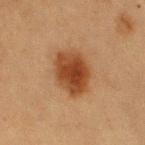Impression:
Recorded during total-body skin imaging; not selected for excision or biopsy.
Clinical summary:
A 15 mm close-up tile from a total-body photography series done for melanoma screening. A female subject aged around 55. The tile uses cross-polarized illumination. The lesion is located on the right upper arm. The lesion's longest dimension is about 5 mm.